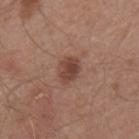Findings:
* biopsy status: imaged on a skin check; not biopsied
* anatomic site: the mid back
* subject: male, aged 63 to 67
* diameter: ≈3 mm
* image source: ~15 mm crop, total-body skin-cancer survey
* tile lighting: white-light illumination